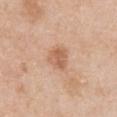Captured during whole-body skin photography for melanoma surveillance; the lesion was not biopsied.